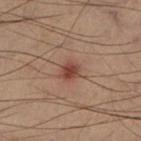<lesion>
  <biopsy_status>not biopsied; imaged during a skin examination</biopsy_status>
  <image>
    <source>total-body photography crop</source>
    <field_of_view_mm>15</field_of_view_mm>
  </image>
  <patient>
    <sex>male</sex>
    <age_approx>55</age_approx>
  </patient>
  <lesion_size>
    <long_diameter_mm_approx>2.5</long_diameter_mm_approx>
  </lesion_size>
  <lighting>cross-polarized</lighting>
  <site>leg</site>
  <automated_metrics>
    <eccentricity>0.5</eccentricity>
    <shape_asymmetry>0.15</shape_asymmetry>
    <nevus_likeness_0_100>90</nevus_likeness_0_100>
    <lesion_detection_confidence_0_100>100</lesion_detection_confidence_0_100>
  </automated_metrics>
</lesion>